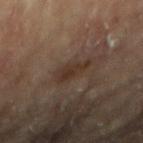Clinical impression:
Captured during whole-body skin photography for melanoma surveillance; the lesion was not biopsied.
Acquisition and patient details:
A female subject, in their 80s. A lesion tile, about 15 mm wide, cut from a 3D total-body photograph. The lesion's longest dimension is about 4 mm. The lesion is on the left arm.